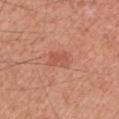Impression:
Captured during whole-body skin photography for melanoma surveillance; the lesion was not biopsied.
Background:
Captured under white-light illumination. About 3 mm across. The lesion is on the left upper arm. An algorithmic analysis of the crop reported a lesion color around L≈55 a*≈29 b*≈32 in CIELAB and a normalized lesion–skin contrast near 5. And it measured border irregularity of about 3 on a 0–10 scale. The analysis additionally found an automated nevus-likeness rating near 0 out of 100 and a detector confidence of about 100 out of 100 that the crop contains a lesion. Cropped from a total-body skin-imaging series; the visible field is about 15 mm. A male patient, aged approximately 60.